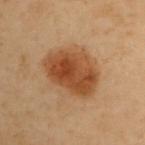| field | value |
|---|---|
| image source | total-body-photography crop, ~15 mm field of view |
| subject | female, aged 58 to 62 |
| lighting | cross-polarized illumination |
| site | the upper back |
| size | about 6.5 mm |